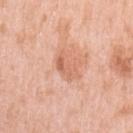Case summary:
* follow-up — imaged on a skin check; not biopsied
* patient — female, roughly 40 years of age
* automated lesion analysis — an outline eccentricity of about 0.85 (0 = round, 1 = elongated) and two-axis asymmetry of about 0.35; an average lesion color of about L≈64 a*≈25 b*≈33 (CIELAB), roughly 9 lightness units darker than nearby skin, and a normalized border contrast of about 5.5
* acquisition — ~15 mm tile from a whole-body skin photo
* lighting — white-light illumination
* lesion diameter — ~3.5 mm (longest diameter)
* body site — the arm